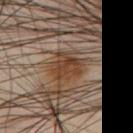Notes:
* notes · total-body-photography surveillance lesion; no biopsy
* illumination · cross-polarized illumination
* image · total-body-photography crop, ~15 mm field of view
* image-analysis metrics · an area of roughly 9.5 mm² and an eccentricity of roughly 0.3; a detector confidence of about 95 out of 100 that the crop contains a lesion
* location · the chest
* patient · male, roughly 45 years of age